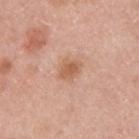| key | value |
|---|---|
| biopsy status | catalogued during a skin exam; not biopsied |
| anatomic site | the upper back |
| illumination | white-light |
| image source | total-body-photography crop, ~15 mm field of view |
| patient | male, roughly 45 years of age |
| size | ≈3 mm |